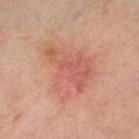Background:
Located on the right leg. Approximately 7 mm at its widest. The patient is a female about 65 years old. Captured under cross-polarized illumination. Cropped from a total-body skin-imaging series; the visible field is about 15 mm.